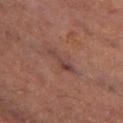This lesion was catalogued during total-body skin photography and was not selected for biopsy. Captured under cross-polarized illumination. Measured at roughly 4 mm in maximum diameter. Cropped from a total-body skin-imaging series; the visible field is about 15 mm. The subject is a male aged 58 to 62. Located on the leg.